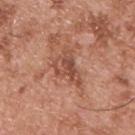  biopsy_status: not biopsied; imaged during a skin examination
  lighting: white-light
  patient:
    sex: male
    age_approx: 55
  lesion_size:
    long_diameter_mm_approx: 5.0
  site: upper back
  image:
    source: total-body photography crop
    field_of_view_mm: 15
  automated_metrics:
    cielab_L: 50
    cielab_a: 24
    cielab_b: 30
    vs_skin_darker_L: 10.0
    vs_skin_contrast_norm: 7.5
    nevus_likeness_0_100: 0